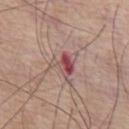Acquisition and patient details:
Longest diameter approximately 3 mm. Located on the chest. A 15 mm crop from a total-body photograph taken for skin-cancer surveillance. The tile uses white-light illumination. A male subject, in their mid-70s. The lesion-visualizer software estimated a footprint of about 5.5 mm² and two-axis asymmetry of about 0.5.